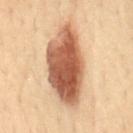notes: no biopsy performed (imaged during a skin exam)
image source: ~15 mm crop, total-body skin-cancer survey
lesion diameter: ≈9 mm
TBP lesion metrics: a peripheral color-asymmetry measure near 2.5
location: the mid back
subject: female, aged 58 to 62
tile lighting: cross-polarized illumination